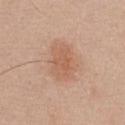notes: no biopsy performed (imaged during a skin exam)
size: ≈5 mm
TBP lesion metrics: a footprint of about 13 mm², an eccentricity of roughly 0.75, and a shape-asymmetry score of about 0.15 (0 = symmetric)
imaging modality: ~15 mm tile from a whole-body skin photo
patient: male, about 30 years old
tile lighting: white-light illumination
anatomic site: the chest